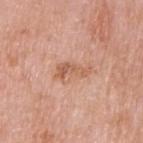Notes:
- follow-up · no biopsy performed (imaged during a skin exam)
- lighting · white-light illumination
- image · ~15 mm crop, total-body skin-cancer survey
- size · ≈4.5 mm
- site · the left upper arm
- subject · male, roughly 60 years of age